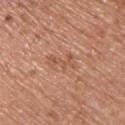Q: Lesion location?
A: the upper back
Q: What are the patient's age and sex?
A: male, aged 53 to 57
Q: How was this image acquired?
A: total-body-photography crop, ~15 mm field of view